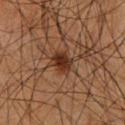Findings:
* biopsy status: no biopsy performed (imaged during a skin exam)
* image source: 15 mm crop, total-body photography
* illumination: cross-polarized
* anatomic site: the chest
* diameter: about 3 mm
* patient: male, roughly 65 years of age
* TBP lesion metrics: a lesion area of about 5.5 mm², an outline eccentricity of about 0.65 (0 = round, 1 = elongated), and a shape-asymmetry score of about 0.25 (0 = symmetric); border irregularity of about 2.5 on a 0–10 scale, a color-variation rating of about 3/10, and a peripheral color-asymmetry measure near 1; an automated nevus-likeness rating near 90 out of 100